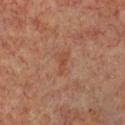Impression:
No biopsy was performed on this lesion — it was imaged during a full skin examination and was not determined to be concerning.
Context:
About 3 mm across. A 15 mm crop from a total-body photograph taken for skin-cancer surveillance. The patient is a female aged around 45. Captured under cross-polarized illumination. On the chest.